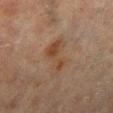Findings:
- automated metrics · a lesion area of about 9 mm², an outline eccentricity of about 0.9 (0 = round, 1 = elongated), and a symmetry-axis asymmetry near 0.4; a detector confidence of about 100 out of 100 that the crop contains a lesion
- site · the left lower leg
- patient · female, in their 80s
- tile lighting · cross-polarized illumination
- imaging modality · 15 mm crop, total-body photography